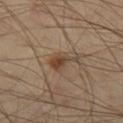<record>
<biopsy_status>not biopsied; imaged during a skin examination</biopsy_status>
<lighting>cross-polarized</lighting>
<site>left thigh</site>
<lesion_size>
  <long_diameter_mm_approx>3.5</long_diameter_mm_approx>
</lesion_size>
<image>
  <source>total-body photography crop</source>
  <field_of_view_mm>15</field_of_view_mm>
</image>
<patient>
  <sex>male</sex>
  <age_approx>40</age_approx>
</patient>
</record>